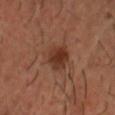No biopsy was performed on this lesion — it was imaged during a full skin examination and was not determined to be concerning.
The tile uses cross-polarized illumination.
The patient is a male approximately 35 years of age.
From the head or neck.
A region of skin cropped from a whole-body photographic capture, roughly 15 mm wide.
Automated image analysis of the tile measured a mean CIELAB color near L≈34 a*≈21 b*≈28, a lesion–skin lightness drop of about 8, and a lesion-to-skin contrast of about 7.5 (normalized; higher = more distinct).
About 4 mm across.